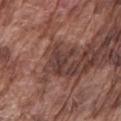This lesion was catalogued during total-body skin photography and was not selected for biopsy. A lesion tile, about 15 mm wide, cut from a 3D total-body photograph. From the left upper arm. The recorded lesion diameter is about 4 mm. This is a white-light tile. A male patient, in their mid-70s.